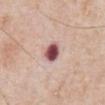This lesion was catalogued during total-body skin photography and was not selected for biopsy. The lesion-visualizer software estimated an eccentricity of roughly 0.45 and a symmetry-axis asymmetry near 0.15. And it measured a mean CIELAB color near L≈51 a*≈26 b*≈19, about 22 CIELAB-L* units darker than the surrounding skin, and a normalized border contrast of about 14. The analysis additionally found an automated nevus-likeness rating near 5 out of 100 and a detector confidence of about 100 out of 100 that the crop contains a lesion. Longest diameter approximately 2.5 mm. A 15 mm close-up tile from a total-body photography series done for melanoma screening. Located on the front of the torso. A male patient, about 80 years old. The tile uses white-light illumination.